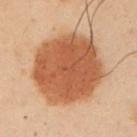Part of a total-body skin-imaging series; this lesion was reviewed on a skin check and was not flagged for biopsy. A male patient aged around 55. About 9.5 mm across. Cropped from a whole-body photographic skin survey; the tile spans about 15 mm. An algorithmic analysis of the crop reported a lesion area of about 48 mm² and a shape-asymmetry score of about 0.1 (0 = symmetric). From the left upper arm.